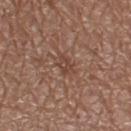Captured during whole-body skin photography for melanoma surveillance; the lesion was not biopsied. Captured under white-light illumination. A lesion tile, about 15 mm wide, cut from a 3D total-body photograph. A female subject approximately 35 years of age. About 2.5 mm across. Located on the right thigh.